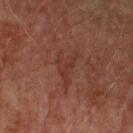image:
  source: total-body photography crop
  field_of_view_mm: 15
site: right forearm
patient:
  sex: male
  age_approx: 75
lesion_size:
  long_diameter_mm_approx: 5.0
lighting: cross-polarized